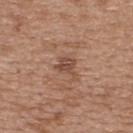biopsy_status: not biopsied; imaged during a skin examination
site: upper back
patient:
  sex: female
  age_approx: 40
lighting: white-light
image:
  source: total-body photography crop
  field_of_view_mm: 15
lesion_size:
  long_diameter_mm_approx: 2.5